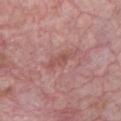Case summary:
– patient · male, approximately 60 years of age
– body site · the chest
– image source · ~15 mm crop, total-body skin-cancer survey